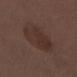The lesion was tiled from a total-body skin photograph and was not biopsied.
About 5.5 mm across.
This is a white-light tile.
A female patient roughly 30 years of age.
An algorithmic analysis of the crop reported an average lesion color of about L≈31 a*≈16 b*≈21 (CIELAB), a lesion–skin lightness drop of about 6, and a normalized border contrast of about 6. The analysis additionally found a border-irregularity index near 2.5/10, a color-variation rating of about 3/10, and peripheral color asymmetry of about 1.
A 15 mm close-up extracted from a 3D total-body photography capture.
The lesion is located on the head or neck.